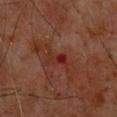This is a cross-polarized tile.
A region of skin cropped from a whole-body photographic capture, roughly 15 mm wide.
The subject is a male aged approximately 60.
The lesion is on the upper back.
The recorded lesion diameter is about 2.5 mm.